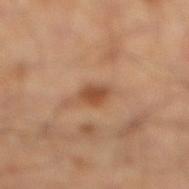Impression:
Recorded during total-body skin imaging; not selected for excision or biopsy.
Image and clinical context:
The subject is a male aged 48–52. A lesion tile, about 15 mm wide, cut from a 3D total-body photograph. The tile uses cross-polarized illumination. The lesion's longest dimension is about 2.5 mm. Automated image analysis of the tile measured internal color variation of about 2 on a 0–10 scale and peripheral color asymmetry of about 0.5. The lesion is on the left leg.